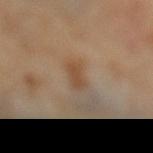This lesion was catalogued during total-body skin photography and was not selected for biopsy.
Approximately 2.5 mm at its widest.
This image is a 15 mm lesion crop taken from a total-body photograph.
From the left lower leg.
A female subject roughly 50 years of age.
This is a cross-polarized tile.
The lesion-visualizer software estimated a footprint of about 4 mm² and an outline eccentricity of about 0.8 (0 = round, 1 = elongated). And it measured a border-irregularity rating of about 3/10, a color-variation rating of about 2.5/10, and radial color variation of about 1. It also reported a lesion-detection confidence of about 100/100.